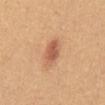Impression: Imaged during a routine full-body skin examination; the lesion was not biopsied and no histopathology is available. Acquisition and patient details: The lesion is on the abdomen. The lesion's longest dimension is about 3.5 mm. The patient is a female aged 23 to 27. The tile uses white-light illumination. Cropped from a whole-body photographic skin survey; the tile spans about 15 mm.